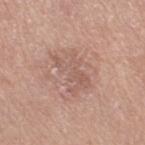Q: Was a biopsy performed?
A: imaged on a skin check; not biopsied
Q: What lighting was used for the tile?
A: white-light illumination
Q: Who is the patient?
A: female, in their mid-50s
Q: Lesion size?
A: about 5 mm
Q: What is the imaging modality?
A: total-body-photography crop, ~15 mm field of view
Q: What did automated image analysis measure?
A: a lesion area of about 10 mm², an outline eccentricity of about 0.8 (0 = round, 1 = elongated), and a shape-asymmetry score of about 0.3 (0 = symmetric)
Q: Lesion location?
A: the leg